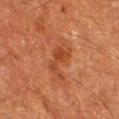follow-up = no biopsy performed (imaged during a skin exam) | site = the right lower leg | tile lighting = cross-polarized illumination | acquisition = total-body-photography crop, ~15 mm field of view | diameter = about 4.5 mm | subject = male, aged approximately 60.